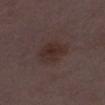Q: Was this lesion biopsied?
A: no biopsy performed (imaged during a skin exam)
Q: How was the tile lit?
A: white-light illumination
Q: What is the anatomic site?
A: the left thigh
Q: What is the imaging modality?
A: ~15 mm crop, total-body skin-cancer survey
Q: What did automated image analysis measure?
A: an area of roughly 9.5 mm² and two-axis asymmetry of about 0.15; a mean CIELAB color near L≈28 a*≈15 b*≈18, roughly 6 lightness units darker than nearby skin, and a normalized lesion–skin contrast near 7.5; a border-irregularity rating of about 1.5/10, a within-lesion color-variation index near 2.5/10, and a peripheral color-asymmetry measure near 1; an automated nevus-likeness rating near 55 out of 100 and a lesion-detection confidence of about 100/100
Q: Patient demographics?
A: female, aged 28 to 32
Q: Lesion size?
A: ~4 mm (longest diameter)